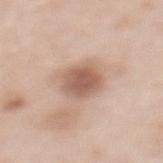Recorded during total-body skin imaging; not selected for excision or biopsy.